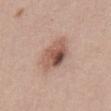| key | value |
|---|---|
| notes | total-body-photography surveillance lesion; no biopsy |
| anatomic site | the abdomen |
| illumination | white-light |
| acquisition | 15 mm crop, total-body photography |
| subject | male, aged approximately 40 |